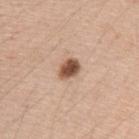Impression: The lesion was tiled from a total-body skin photograph and was not biopsied. Background: The patient is a female aged 43–47. A roughly 15 mm field-of-view crop from a total-body skin photograph. The tile uses white-light illumination. The total-body-photography lesion software estimated a nevus-likeness score of about 100/100 and a lesion-detection confidence of about 100/100. Measured at roughly 3 mm in maximum diameter. The lesion is located on the left upper arm.